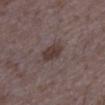The lesion is located on the right lower leg. The patient is a female in their mid-30s. A 15 mm crop from a total-body photograph taken for skin-cancer surveillance.